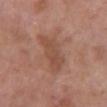Impression: This lesion was catalogued during total-body skin photography and was not selected for biopsy. Clinical summary: The subject is a female aged 68–72. From the chest. An algorithmic analysis of the crop reported a lesion color around L≈49 a*≈21 b*≈28 in CIELAB, about 7 CIELAB-L* units darker than the surrounding skin, and a lesion-to-skin contrast of about 5.5 (normalized; higher = more distinct). And it measured border irregularity of about 4.5 on a 0–10 scale, internal color variation of about 2 on a 0–10 scale, and peripheral color asymmetry of about 0.5. A region of skin cropped from a whole-body photographic capture, roughly 15 mm wide.